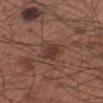Q: Lesion location?
A: the right forearm
Q: Patient demographics?
A: male, aged around 55
Q: What lighting was used for the tile?
A: white-light
Q: Lesion size?
A: ≈2.5 mm
Q: What is the imaging modality?
A: total-body-photography crop, ~15 mm field of view